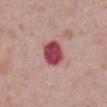biopsy_status: not biopsied; imaged during a skin examination
image:
  source: total-body photography crop
  field_of_view_mm: 15
patient:
  sex: male
  age_approx: 60
site: front of the torso